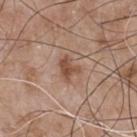workup: no biopsy performed (imaged during a skin exam) | location: the chest | subject: male, aged 63 to 67 | image source: ~15 mm crop, total-body skin-cancer survey | size: about 2.5 mm | tile lighting: white-light.